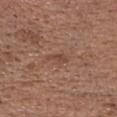  biopsy_status: not biopsied; imaged during a skin examination
  image:
    source: total-body photography crop
    field_of_view_mm: 15
  patient:
    sex: male
    age_approx: 75
  lighting: white-light
  lesion_size:
    long_diameter_mm_approx: 3.0
  site: head or neck
  automated_metrics:
    area_mm2_approx: 3.0
    shape_asymmetry: 0.4
    cielab_L: 45
    cielab_a: 20
    cielab_b: 27
    vs_skin_darker_L: 7.0
    vs_skin_contrast_norm: 5.5